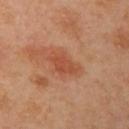The lesion was tiled from a total-body skin photograph and was not biopsied. Located on the left upper arm. Captured under cross-polarized illumination. A female patient aged approximately 40. Approximately 3.5 mm at its widest. A close-up tile cropped from a whole-body skin photograph, about 15 mm across. Automated tile analysis of the lesion measured a mean CIELAB color near L≈51 a*≈28 b*≈36, about 8 CIELAB-L* units darker than the surrounding skin, and a normalized lesion–skin contrast near 6.